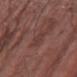Q: Was this lesion biopsied?
A: total-body-photography surveillance lesion; no biopsy
Q: Patient demographics?
A: male, in their mid-70s
Q: Automated lesion metrics?
A: a footprint of about 4 mm² and a shape eccentricity near 0.85; about 5 CIELAB-L* units darker than the surrounding skin and a normalized border contrast of about 5; a within-lesion color-variation index near 2/10; a detector confidence of about 100 out of 100 that the crop contains a lesion
Q: What is the lesion's diameter?
A: ≈3 mm
Q: Illumination type?
A: white-light illumination
Q: Where on the body is the lesion?
A: the left forearm
Q: What kind of image is this?
A: ~15 mm tile from a whole-body skin photo